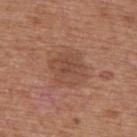follow-up = imaged on a skin check; not biopsied | automated metrics = a footprint of about 15 mm², an eccentricity of roughly 0.4, and a symmetry-axis asymmetry near 0.15; a border-irregularity rating of about 2/10, a within-lesion color-variation index near 3/10, and a peripheral color-asymmetry measure near 1; a classifier nevus-likeness of about 10/100 | location = the upper back | subject = male, aged 63–67 | image source = ~15 mm crop, total-body skin-cancer survey | illumination = white-light | lesion diameter = ≈4.5 mm.